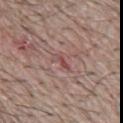Q: Is there a histopathology result?
A: catalogued during a skin exam; not biopsied
Q: How large is the lesion?
A: about 3 mm
Q: Where on the body is the lesion?
A: the mid back
Q: How was the tile lit?
A: white-light illumination
Q: What are the patient's age and sex?
A: male, aged around 65
Q: What is the imaging modality?
A: ~15 mm tile from a whole-body skin photo